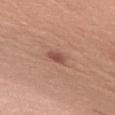Case summary:
- anatomic site: the upper back
- image: total-body-photography crop, ~15 mm field of view
- subject: female, roughly 40 years of age
- TBP lesion metrics: a lesion color around L≈53 a*≈23 b*≈28 in CIELAB, roughly 9 lightness units darker than nearby skin, and a normalized lesion–skin contrast near 6
- lesion size: about 3 mm
- lighting: white-light illumination